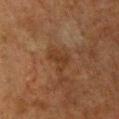<case>
<biopsy_status>not biopsied; imaged during a skin examination</biopsy_status>
<lighting>cross-polarized</lighting>
<site>chest</site>
<automated_metrics>
  <vs_skin_darker_L>6.0</vs_skin_darker_L>
  <vs_skin_contrast_norm>6.0</vs_skin_contrast_norm>
</automated_metrics>
<patient>
  <sex>female</sex>
  <age_approx>55</age_approx>
</patient>
<lesion_size>
  <long_diameter_mm_approx>3.0</long_diameter_mm_approx>
</lesion_size>
<image>
  <source>total-body photography crop</source>
  <field_of_view_mm>15</field_of_view_mm>
</image>
</case>